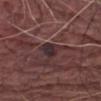Clinical impression:
The lesion was photographed on a routine skin check and not biopsied; there is no pathology result.
Background:
A close-up tile cropped from a whole-body skin photograph, about 15 mm across. Imaged with white-light lighting. On the left thigh. The recorded lesion diameter is about 3 mm. The subject is a male approximately 80 years of age.